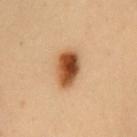Q: Was this lesion biopsied?
A: catalogued during a skin exam; not biopsied
Q: Lesion location?
A: the chest
Q: How large is the lesion?
A: ≈4.5 mm
Q: Automated lesion metrics?
A: roughly 17 lightness units darker than nearby skin; a border-irregularity rating of about 2/10, internal color variation of about 8 on a 0–10 scale, and peripheral color asymmetry of about 2.5
Q: How was the tile lit?
A: cross-polarized illumination
Q: Patient demographics?
A: female, aged 48 to 52
Q: What kind of image is this?
A: ~15 mm tile from a whole-body skin photo